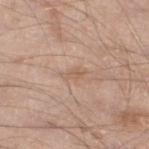Q: What is the imaging modality?
A: ~15 mm crop, total-body skin-cancer survey
Q: How was the tile lit?
A: white-light
Q: What is the lesion's diameter?
A: ~2.5 mm (longest diameter)
Q: Who is the patient?
A: male, aged approximately 60
Q: Lesion location?
A: the leg
Q: What did automated image analysis measure?
A: an average lesion color of about L≈59 a*≈18 b*≈30 (CIELAB), roughly 7 lightness units darker than nearby skin, and a normalized lesion–skin contrast near 5; a classifier nevus-likeness of about 0/100 and lesion-presence confidence of about 100/100